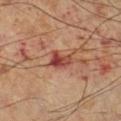Recorded during total-body skin imaging; not selected for excision or biopsy. A male patient approximately 60 years of age. The lesion is on the left lower leg. A 15 mm close-up extracted from a 3D total-body photography capture. The tile uses cross-polarized illumination. Approximately 3 mm at its widest.